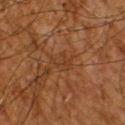Recorded during total-body skin imaging; not selected for excision or biopsy.